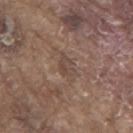Q: Was a biopsy performed?
A: no biopsy performed (imaged during a skin exam)
Q: What is the anatomic site?
A: the mid back
Q: How was this image acquired?
A: total-body-photography crop, ~15 mm field of view
Q: Patient demographics?
A: male, aged 78 to 82